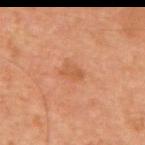Imaged during a routine full-body skin examination; the lesion was not biopsied and no histopathology is available. An algorithmic analysis of the crop reported an average lesion color of about L≈50 a*≈24 b*≈35 (CIELAB) and a lesion-to-skin contrast of about 5.5 (normalized; higher = more distinct). And it measured a border-irregularity index near 2.5/10 and peripheral color asymmetry of about 0.5. The analysis additionally found a nevus-likeness score of about 5/100 and lesion-presence confidence of about 100/100. The lesion is on the chest. Cropped from a total-body skin-imaging series; the visible field is about 15 mm. Approximately 2.5 mm at its widest. A male subject, in their 60s.